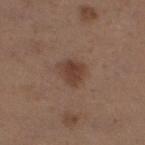* workup · imaged on a skin check; not biopsied
* location · the right thigh
* subject · female, in their 30s
* acquisition · ~15 mm tile from a whole-body skin photo